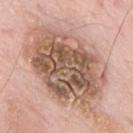Case summary:
* workup · imaged on a skin check; not biopsied
* illumination · white-light
* imaging modality · 15 mm crop, total-body photography
* subject · male, roughly 60 years of age
* lesion diameter · ~11 mm (longest diameter)
* image-analysis metrics · an eccentricity of roughly 0.75 and a symmetry-axis asymmetry near 0.2; roughly 15 lightness units darker than nearby skin and a normalized border contrast of about 9.5; border irregularity of about 4.5 on a 0–10 scale, a within-lesion color-variation index near 8.5/10, and peripheral color asymmetry of about 3
* anatomic site · the mid back